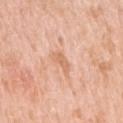biopsy_status: not biopsied; imaged during a skin examination
lesion_size:
  long_diameter_mm_approx: 3.0
site: right upper arm
image:
  source: total-body photography crop
  field_of_view_mm: 15
automated_metrics:
  eccentricity: 0.85
  shape_asymmetry: 0.4
  cielab_L: 67
  cielab_a: 23
  cielab_b: 35
  vs_skin_contrast_norm: 5.5
  border_irregularity_0_10: 4.5
  color_variation_0_10: 1.0
lighting: white-light
patient:
  sex: female
  age_approx: 40